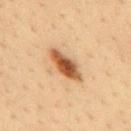workup — imaged on a skin check; not biopsied | image source — ~15 mm crop, total-body skin-cancer survey | image-analysis metrics — a lesion area of about 8 mm², an eccentricity of roughly 0.9, and a shape-asymmetry score of about 0.25 (0 = symmetric); a normalized border contrast of about 11; a detector confidence of about 100 out of 100 that the crop contains a lesion | patient — male, aged approximately 35 | site — the mid back | size — about 5 mm.